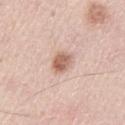Case summary:
• location — the left upper arm
• lesion diameter — about 3 mm
• subject — male, aged around 45
• image — 15 mm crop, total-body photography
• automated lesion analysis — a border-irregularity rating of about 2/10, a within-lesion color-variation index near 3.5/10, and radial color variation of about 1.5